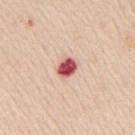Imaged during a routine full-body skin examination; the lesion was not biopsied and no histopathology is available. This is a white-light tile. On the mid back. About 2.5 mm across. A male subject in their mid- to late 60s. A 15 mm close-up extracted from a 3D total-body photography capture.